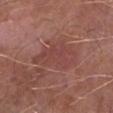Captured during whole-body skin photography for melanoma surveillance; the lesion was not biopsied.
The lesion's longest dimension is about 6 mm.
A male patient aged 63–67.
The lesion is located on the left lower leg.
Cropped from a total-body skin-imaging series; the visible field is about 15 mm.
Imaged with white-light lighting.